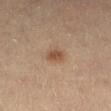A female subject, roughly 30 years of age. The lesion is located on the left lower leg. Cropped from a total-body skin-imaging series; the visible field is about 15 mm.